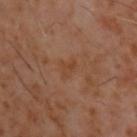| key | value |
|---|---|
| workup | catalogued during a skin exam; not biopsied |
| size | ~2.5 mm (longest diameter) |
| illumination | cross-polarized illumination |
| body site | the back |
| image-analysis metrics | an area of roughly 3 mm², an eccentricity of roughly 0.75, and a shape-asymmetry score of about 0.45 (0 = symmetric); a classifier nevus-likeness of about 0/100 and a lesion-detection confidence of about 100/100 |
| acquisition | ~15 mm tile from a whole-body skin photo |
| subject | male, aged approximately 60 |